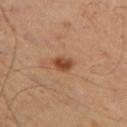Q: Was a biopsy performed?
A: catalogued during a skin exam; not biopsied
Q: Illumination type?
A: cross-polarized
Q: What are the patient's age and sex?
A: male, aged around 55
Q: Where on the body is the lesion?
A: the left thigh
Q: How was this image acquired?
A: total-body-photography crop, ~15 mm field of view